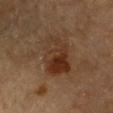Q: Is there a histopathology result?
A: no biopsy performed (imaged during a skin exam)
Q: Where on the body is the lesion?
A: the chest
Q: What are the patient's age and sex?
A: male, aged 83 or older
Q: How was this image acquired?
A: ~15 mm tile from a whole-body skin photo
Q: How large is the lesion?
A: ≈6 mm
Q: Automated lesion metrics?
A: internal color variation of about 8 on a 0–10 scale